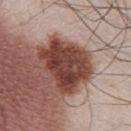Recorded during total-body skin imaging; not selected for excision or biopsy.
A male patient, about 45 years old.
On the front of the torso.
Automated tile analysis of the lesion measured an average lesion color of about L≈41 a*≈24 b*≈25 (CIELAB), roughly 17 lightness units darker than nearby skin, and a normalized border contrast of about 12.5. And it measured a nevus-likeness score of about 35/100 and a detector confidence of about 100 out of 100 that the crop contains a lesion.
The recorded lesion diameter is about 6.5 mm.
A close-up tile cropped from a whole-body skin photograph, about 15 mm across.